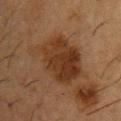<lesion>
<biopsy_status>not biopsied; imaged during a skin examination</biopsy_status>
<lesion_size>
  <long_diameter_mm_approx>7.0</long_diameter_mm_approx>
</lesion_size>
<lighting>cross-polarized</lighting>
<patient>
  <sex>male</sex>
  <age_approx>55</age_approx>
</patient>
<image>
  <source>total-body photography crop</source>
  <field_of_view_mm>15</field_of_view_mm>
</image>
<site>upper back</site>
</lesion>